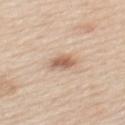{"site": "back", "patient": {"sex": "male", "age_approx": 60}, "image": {"source": "total-body photography crop", "field_of_view_mm": 15}}